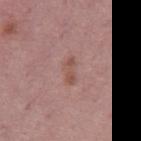Impression: The lesion was tiled from a total-body skin photograph and was not biopsied. Context: A region of skin cropped from a whole-body photographic capture, roughly 15 mm wide. Located on the right thigh. A female subject aged approximately 50.